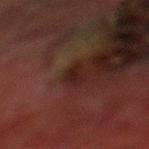Imaged during a routine full-body skin examination; the lesion was not biopsied and no histopathology is available.
On the leg.
A male subject, aged around 70.
A 15 mm close-up tile from a total-body photography series done for melanoma screening.
This is a cross-polarized tile.
Measured at roughly 2.5 mm in maximum diameter.
Automated image analysis of the tile measured a shape-asymmetry score of about 0.35 (0 = symmetric). The analysis additionally found an average lesion color of about L≈16 a*≈17 b*≈18 (CIELAB) and a normalized lesion–skin contrast near 7. It also reported a border-irregularity index near 3.5/10, a color-variation rating of about 0/10, and a peripheral color-asymmetry measure near 0. The analysis additionally found an automated nevus-likeness rating near 5 out of 100 and a detector confidence of about 80 out of 100 that the crop contains a lesion.